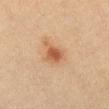No biopsy was performed on this lesion — it was imaged during a full skin examination and was not determined to be concerning. A female patient aged around 40. A roughly 15 mm field-of-view crop from a total-body skin photograph. Measured at roughly 2.5 mm in maximum diameter. The lesion is located on the abdomen. Automated image analysis of the tile measured a lesion area of about 4 mm² and a shape-asymmetry score of about 0.1 (0 = symmetric). And it measured a border-irregularity rating of about 1/10 and a peripheral color-asymmetry measure near 1.